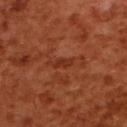| key | value |
|---|---|
| notes | catalogued during a skin exam; not biopsied |
| diameter | ~2.5 mm (longest diameter) |
| patient | female, aged approximately 55 |
| lighting | cross-polarized illumination |
| location | the upper back |
| image | ~15 mm tile from a whole-body skin photo |